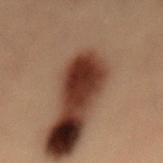Part of a total-body skin-imaging series; this lesion was reviewed on a skin check and was not flagged for biopsy.
A 15 mm crop from a total-body photograph taken for skin-cancer surveillance.
This is a cross-polarized tile.
The patient is a male approximately 55 years of age.
On the lower back.